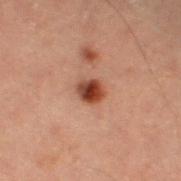Clinical impression: Recorded during total-body skin imaging; not selected for excision or biopsy. Image and clinical context: Measured at roughly 2.5 mm in maximum diameter. A roughly 15 mm field-of-view crop from a total-body skin photograph. A male subject aged approximately 60. An algorithmic analysis of the crop reported about 13 CIELAB-L* units darker than the surrounding skin. And it measured a border-irregularity rating of about 1.5/10, a color-variation rating of about 6.5/10, and peripheral color asymmetry of about 2. The tile uses cross-polarized illumination. On the left thigh.